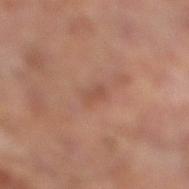{"biopsy_status": "not biopsied; imaged during a skin examination", "automated_metrics": {"area_mm2_approx": 3.5, "shape_asymmetry": 0.25, "border_irregularity_0_10": 2.5, "color_variation_0_10": 2.5, "peripheral_color_asymmetry": 1.0, "lesion_detection_confidence_0_100": 100}, "site": "right lower leg", "image": {"source": "total-body photography crop", "field_of_view_mm": 15}, "patient": {"sex": "male", "age_approx": 65}, "lesion_size": {"long_diameter_mm_approx": 2.5}}